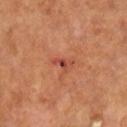Assessment:
Imaged during a routine full-body skin examination; the lesion was not biopsied and no histopathology is available.
Background:
A lesion tile, about 15 mm wide, cut from a 3D total-body photograph. The lesion-visualizer software estimated a footprint of about 2.5 mm², a shape eccentricity near 0.85, and a symmetry-axis asymmetry near 0.5. The software also gave a nevus-likeness score of about 0/100 and lesion-presence confidence of about 100/100. Captured under cross-polarized illumination. A male subject aged around 65. The lesion is located on the left leg.